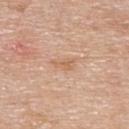The lesion was photographed on a routine skin check and not biopsied; there is no pathology result. A 15 mm close-up extracted from a 3D total-body photography capture. A male subject, roughly 55 years of age. On the upper back. Imaged with white-light lighting. About 3 mm across. An algorithmic analysis of the crop reported a lesion area of about 3.5 mm² and an outline eccentricity of about 0.85 (0 = round, 1 = elongated). The analysis additionally found an average lesion color of about L≈63 a*≈20 b*≈34 (CIELAB), about 7 CIELAB-L* units darker than the surrounding skin, and a normalized lesion–skin contrast near 5.5. It also reported border irregularity of about 4.5 on a 0–10 scale, internal color variation of about 2 on a 0–10 scale, and a peripheral color-asymmetry measure near 1.